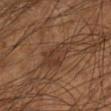Imaged during a routine full-body skin examination; the lesion was not biopsied and no histopathology is available.
Approximately 5 mm at its widest.
From the left lower leg.
This image is a 15 mm lesion crop taken from a total-body photograph.
The patient is a male approximately 55 years of age.
An algorithmic analysis of the crop reported a shape eccentricity near 0.85 and a shape-asymmetry score of about 0.3 (0 = symmetric). The software also gave border irregularity of about 3.5 on a 0–10 scale, internal color variation of about 3 on a 0–10 scale, and a peripheral color-asymmetry measure near 1. It also reported an automated nevus-likeness rating near 0 out of 100.
Imaged with cross-polarized lighting.